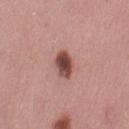The lesion was photographed on a routine skin check and not biopsied; there is no pathology result. Automated image analysis of the tile measured an outline eccentricity of about 0.65 (0 = round, 1 = elongated) and a shape-asymmetry score of about 0.15 (0 = symmetric). The software also gave an average lesion color of about L≈48 a*≈23 b*≈23 (CIELAB) and about 16 CIELAB-L* units darker than the surrounding skin. The analysis additionally found a border-irregularity rating of about 1.5/10 and a color-variation rating of about 6/10. The analysis additionally found an automated nevus-likeness rating near 95 out of 100 and a lesion-detection confidence of about 100/100. This is a white-light tile. The lesion's longest dimension is about 3.5 mm. Located on the leg. A female patient about 30 years old. This image is a 15 mm lesion crop taken from a total-body photograph.